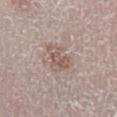Q: Patient demographics?
A: female, about 30 years old
Q: How was this image acquired?
A: total-body-photography crop, ~15 mm field of view
Q: Where on the body is the lesion?
A: the left lower leg
Q: What lighting was used for the tile?
A: white-light
Q: Lesion size?
A: about 3.5 mm
Q: Automated lesion metrics?
A: a mean CIELAB color near L≈56 a*≈15 b*≈23; border irregularity of about 4 on a 0–10 scale, internal color variation of about 3.5 on a 0–10 scale, and a peripheral color-asymmetry measure near 1; a lesion-detection confidence of about 90/100